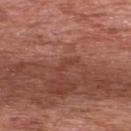This lesion was catalogued during total-body skin photography and was not selected for biopsy. A close-up tile cropped from a whole-body skin photograph, about 15 mm across. From the upper back. The patient is a male aged 68 to 72.